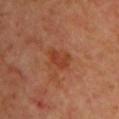{
  "biopsy_status": "not biopsied; imaged during a skin examination",
  "image": {
    "source": "total-body photography crop",
    "field_of_view_mm": 15
  },
  "lesion_size": {
    "long_diameter_mm_approx": 3.0
  },
  "site": "chest",
  "patient": {
    "sex": "female",
    "age_approx": 40
  }
}